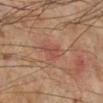No biopsy was performed on this lesion — it was imaged during a full skin examination and was not determined to be concerning.
Imaged with cross-polarized lighting.
A region of skin cropped from a whole-body photographic capture, roughly 15 mm wide.
From the right lower leg.
The lesion's longest dimension is about 2.5 mm.
The patient is a male in their 60s.